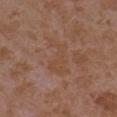Findings:
• follow-up — catalogued during a skin exam; not biopsied
• patient — female, about 35 years old
• tile lighting — white-light
• image — total-body-photography crop, ~15 mm field of view
• body site — the left upper arm
• diameter — ~5 mm (longest diameter)